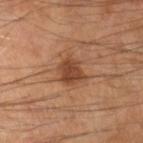– notes — catalogued during a skin exam; not biopsied
– location — the right forearm
– imaging modality — ~15 mm crop, total-body skin-cancer survey
– TBP lesion metrics — a border-irregularity rating of about 2.5/10, a color-variation rating of about 2.5/10, and a peripheral color-asymmetry measure near 1
– subject — male, about 70 years old